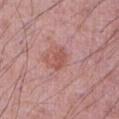Imaged during a routine full-body skin examination; the lesion was not biopsied and no histopathology is available. Longest diameter approximately 3 mm. Located on the abdomen. The subject is a male roughly 70 years of age. Cropped from a total-body skin-imaging series; the visible field is about 15 mm. The total-body-photography lesion software estimated a lesion color around L≈53 a*≈26 b*≈25 in CIELAB, about 8 CIELAB-L* units darker than the surrounding skin, and a normalized lesion–skin contrast near 6. And it measured an automated nevus-likeness rating near 5 out of 100.